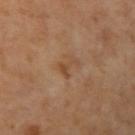Q: Was this lesion biopsied?
A: total-body-photography surveillance lesion; no biopsy
Q: What is the imaging modality?
A: ~15 mm crop, total-body skin-cancer survey
Q: Automated lesion metrics?
A: an area of roughly 3 mm², an outline eccentricity of about 0.85 (0 = round, 1 = elongated), and two-axis asymmetry of about 0.6; a mean CIELAB color near L≈48 a*≈20 b*≈34, roughly 7 lightness units darker than nearby skin, and a normalized lesion–skin contrast near 5.5; a border-irregularity rating of about 6.5/10 and a color-variation rating of about 0.5/10; an automated nevus-likeness rating near 0 out of 100 and lesion-presence confidence of about 100/100
Q: Illumination type?
A: cross-polarized illumination
Q: What are the patient's age and sex?
A: female, roughly 60 years of age
Q: Lesion location?
A: the arm